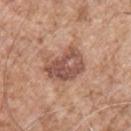The lesion was tiled from a total-body skin photograph and was not biopsied. Cropped from a total-body skin-imaging series; the visible field is about 15 mm. Captured under white-light illumination. On the chest. Measured at roughly 5 mm in maximum diameter. A male patient, about 55 years old. The total-body-photography lesion software estimated border irregularity of about 3.5 on a 0–10 scale and radial color variation of about 2.5. It also reported an automated nevus-likeness rating near 25 out of 100 and lesion-presence confidence of about 100/100.